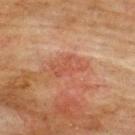From the upper back.
Approximately 4 mm at its widest.
A male patient in their mid- to late 70s.
A 15 mm crop from a total-body photograph taken for skin-cancer surveillance.
The tile uses cross-polarized illumination.